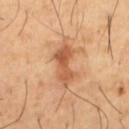The lesion was photographed on a routine skin check and not biopsied; there is no pathology result.
A 15 mm close-up extracted from a 3D total-body photography capture.
A male subject approximately 65 years of age.
Approximately 5 mm at its widest.
On the left thigh.
The tile uses cross-polarized illumination.
Automated image analysis of the tile measured a lesion area of about 9 mm² and a symmetry-axis asymmetry near 0.3. It also reported a lesion color around L≈57 a*≈24 b*≈37 in CIELAB. And it measured border irregularity of about 4 on a 0–10 scale and a peripheral color-asymmetry measure near 2. It also reported an automated nevus-likeness rating near 0 out of 100.